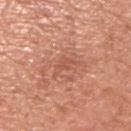Imaged during a routine full-body skin examination; the lesion was not biopsied and no histopathology is available. The lesion-visualizer software estimated an area of roughly 3.5 mm² and an outline eccentricity of about 0.8 (0 = round, 1 = elongated). The software also gave a border-irregularity index near 6/10, a within-lesion color-variation index near 1/10, and peripheral color asymmetry of about 0.5. The software also gave an automated nevus-likeness rating near 0 out of 100 and a lesion-detection confidence of about 100/100. A male subject aged approximately 65. Approximately 3.5 mm at its widest. A 15 mm close-up extracted from a 3D total-body photography capture. The lesion is on the right upper arm.